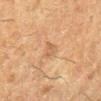Clinical impression:
Imaged during a routine full-body skin examination; the lesion was not biopsied and no histopathology is available.
Background:
Imaged with cross-polarized lighting. Cropped from a whole-body photographic skin survey; the tile spans about 15 mm. Automated image analysis of the tile measured a lesion color around L≈49 a*≈18 b*≈31 in CIELAB and a lesion-to-skin contrast of about 4.5 (normalized; higher = more distinct). The analysis additionally found a border-irregularity index near 3/10 and internal color variation of about 2 on a 0–10 scale. And it measured an automated nevus-likeness rating near 0 out of 100 and a lesion-detection confidence of about 100/100. A female subject about 50 years old. The lesion is located on the right lower leg.